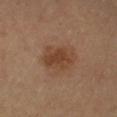illumination — cross-polarized illumination | patient — male, aged around 55 | lesion diameter — ≈4.5 mm | anatomic site — the right forearm | image — total-body-photography crop, ~15 mm field of view | automated lesion analysis — a lesion-to-skin contrast of about 7.5 (normalized; higher = more distinct); an automated nevus-likeness rating near 80 out of 100.